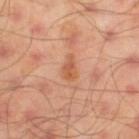Context:
About 3 mm across. A close-up tile cropped from a whole-body skin photograph, about 15 mm across. A male subject, aged around 45. Located on the left thigh. Captured under cross-polarized illumination. An algorithmic analysis of the crop reported a border-irregularity rating of about 3/10, a within-lesion color-variation index near 2.5/10, and a peripheral color-asymmetry measure near 1.